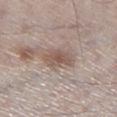A male subject, aged around 60. A 15 mm close-up extracted from a 3D total-body photography capture. From the right lower leg. This is a white-light tile. The lesion-visualizer software estimated roughly 9 lightness units darker than nearby skin. The recorded lesion diameter is about 4 mm.